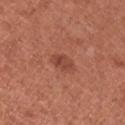workup = no biopsy performed (imaged during a skin exam); imaging modality = ~15 mm crop, total-body skin-cancer survey; lighting = white-light; patient = female, aged around 50; lesion size = about 3 mm; anatomic site = the right upper arm.